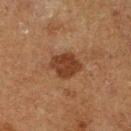Q: Was this lesion biopsied?
A: no biopsy performed (imaged during a skin exam)
Q: What lighting was used for the tile?
A: cross-polarized illumination
Q: How large is the lesion?
A: about 4 mm
Q: Where on the body is the lesion?
A: the right lower leg
Q: What are the patient's age and sex?
A: male, in their mid-70s
Q: What kind of image is this?
A: ~15 mm tile from a whole-body skin photo
Q: What did automated image analysis measure?
A: a nevus-likeness score of about 70/100 and a detector confidence of about 100 out of 100 that the crop contains a lesion